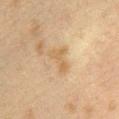{"biopsy_status": "not biopsied; imaged during a skin examination", "lighting": "cross-polarized", "automated_metrics": {"area_mm2_approx": 5.5, "eccentricity": 0.8, "shape_asymmetry": 0.55, "cielab_L": 52, "cielab_a": 13, "cielab_b": 33, "vs_skin_darker_L": 6.0, "vs_skin_contrast_norm": 6.0, "border_irregularity_0_10": 6.0, "peripheral_color_asymmetry": 0.5}, "site": "arm", "patient": {"sex": "female", "age_approx": 40}, "lesion_size": {"long_diameter_mm_approx": 3.5}, "image": {"source": "total-body photography crop", "field_of_view_mm": 15}}